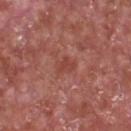The lesion was tiled from a total-body skin photograph and was not biopsied. The lesion-visualizer software estimated a lesion area of about 4 mm² and two-axis asymmetry of about 0.3. A male patient approximately 65 years of age. This image is a 15 mm lesion crop taken from a total-body photograph. About 2.5 mm across. The lesion is on the chest.